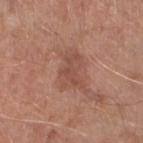{
  "biopsy_status": "not biopsied; imaged during a skin examination",
  "lesion_size": {
    "long_diameter_mm_approx": 4.0
  },
  "image": {
    "source": "total-body photography crop",
    "field_of_view_mm": 15
  },
  "site": "right lower leg",
  "patient": {
    "sex": "male",
    "age_approx": 65
  },
  "lighting": "white-light"
}